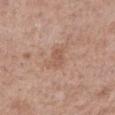– TBP lesion metrics — a normalized border contrast of about 5; a border-irregularity index near 3.5/10, a color-variation rating of about 0/10, and peripheral color asymmetry of about 0; a classifier nevus-likeness of about 0/100 and lesion-presence confidence of about 100/100
– size — ~2.5 mm (longest diameter)
– patient — female, in their 40s
– tile lighting — white-light illumination
– location — the right forearm
– acquisition — 15 mm crop, total-body photography